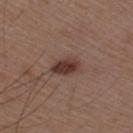Assessment:
Imaged during a routine full-body skin examination; the lesion was not biopsied and no histopathology is available.
Background:
Automated image analysis of the tile measured an area of roughly 5.5 mm² and a shape eccentricity near 0.8. And it measured a lesion color around L≈36 a*≈19 b*≈23 in CIELAB, a lesion–skin lightness drop of about 12, and a normalized lesion–skin contrast near 10. The software also gave a border-irregularity index near 2.5/10, internal color variation of about 4 on a 0–10 scale, and radial color variation of about 1.5. It also reported a classifier nevus-likeness of about 95/100 and a detector confidence of about 100 out of 100 that the crop contains a lesion. From the arm. A close-up tile cropped from a whole-body skin photograph, about 15 mm across. A male patient, approximately 50 years of age. Imaged with white-light lighting.